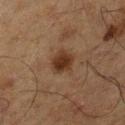<case>
  <site>right lower leg</site>
  <image>
    <source>total-body photography crop</source>
    <field_of_view_mm>15</field_of_view_mm>
  </image>
  <patient>
    <sex>male</sex>
    <age_approx>65</age_approx>
  </patient>
  <lighting>cross-polarized</lighting>
  <lesion_size>
    <long_diameter_mm_approx>3.0</long_diameter_mm_approx>
  </lesion_size>
</case>